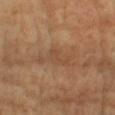This lesion was catalogued during total-body skin photography and was not selected for biopsy. This is a cross-polarized tile. A patient about 60 years old. Longest diameter approximately 3 mm. Automated tile analysis of the lesion measured a lesion area of about 3.5 mm², an outline eccentricity of about 0.9 (0 = round, 1 = elongated), and two-axis asymmetry of about 0.55. The analysis additionally found a border-irregularity index near 6/10, a within-lesion color-variation index near 1.5/10, and peripheral color asymmetry of about 0.5. This image is a 15 mm lesion crop taken from a total-body photograph. From the chest.